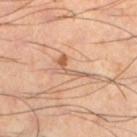Impression: Recorded during total-body skin imaging; not selected for excision or biopsy. Acquisition and patient details: A male subject, aged 38–42. The lesion is located on the left lower leg. This is a cross-polarized tile. A 15 mm crop from a total-body photograph taken for skin-cancer surveillance.